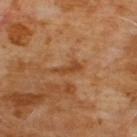{
  "biopsy_status": "not biopsied; imaged during a skin examination",
  "lesion_size": {
    "long_diameter_mm_approx": 3.5
  },
  "patient": {
    "sex": "male",
    "age_approx": 60
  },
  "site": "chest",
  "image": {
    "source": "total-body photography crop",
    "field_of_view_mm": 15
  }
}